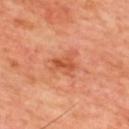biopsy_status: not biopsied; imaged during a skin examination
patient:
  sex: male
  age_approx: 60
lighting: cross-polarized
image:
  source: total-body photography crop
  field_of_view_mm: 15
automated_metrics:
  nevus_likeness_0_100: 5
  lesion_detection_confidence_0_100: 100
site: upper back
lesion_size:
  long_diameter_mm_approx: 2.5